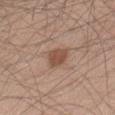Clinical impression: Imaged during a routine full-body skin examination; the lesion was not biopsied and no histopathology is available. Background: The total-body-photography lesion software estimated an area of roughly 5.5 mm². It also reported a lesion color around L≈50 a*≈19 b*≈28 in CIELAB. The analysis additionally found a color-variation rating of about 2.5/10 and a peripheral color-asymmetry measure near 1. It also reported a nevus-likeness score of about 90/100 and a detector confidence of about 100 out of 100 that the crop contains a lesion. The tile uses white-light illumination. The lesion is located on the leg. A 15 mm close-up tile from a total-body photography series done for melanoma screening. The recorded lesion diameter is about 3 mm. The patient is a male in their 60s.